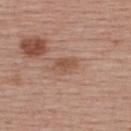Background: Located on the upper back. Captured under white-light illumination. A close-up tile cropped from a whole-body skin photograph, about 15 mm across. Measured at roughly 3 mm in maximum diameter. The total-body-photography lesion software estimated a lesion area of about 5 mm², an eccentricity of roughly 0.8, and a shape-asymmetry score of about 0.25 (0 = symmetric). The software also gave internal color variation of about 2.5 on a 0–10 scale and peripheral color asymmetry of about 1. A female subject, about 55 years old.